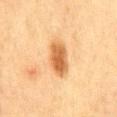{"biopsy_status": "not biopsied; imaged during a skin examination", "site": "mid back", "lesion_size": {"long_diameter_mm_approx": 5.0}, "automated_metrics": {"eccentricity": 0.9, "shape_asymmetry": 0.2, "cielab_L": 53, "cielab_a": 22, "cielab_b": 39, "vs_skin_darker_L": 14.0, "vs_skin_contrast_norm": 9.5}, "image": {"source": "total-body photography crop", "field_of_view_mm": 15}, "lighting": "cross-polarized", "patient": {"sex": "female", "age_approx": 55}}